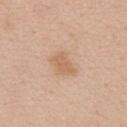This lesion was catalogued during total-body skin photography and was not selected for biopsy.
A female subject, aged 43 to 47.
A close-up tile cropped from a whole-body skin photograph, about 15 mm across.
Longest diameter approximately 3 mm.
The lesion-visualizer software estimated a footprint of about 4.5 mm², an eccentricity of roughly 0.8, and a shape-asymmetry score of about 0.3 (0 = symmetric). And it measured border irregularity of about 3 on a 0–10 scale, a color-variation rating of about 1.5/10, and a peripheral color-asymmetry measure near 0.5.
On the chest.